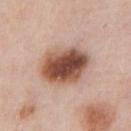Assessment:
No biopsy was performed on this lesion — it was imaged during a full skin examination and was not determined to be concerning.
Acquisition and patient details:
Captured under white-light illumination. The lesion's longest dimension is about 6 mm. Automated image analysis of the tile measured an eccentricity of roughly 0.65 and two-axis asymmetry of about 0.1. The analysis additionally found an automated nevus-likeness rating near 100 out of 100 and a detector confidence of about 100 out of 100 that the crop contains a lesion. Located on the front of the torso. A male patient in their mid- to late 50s. A 15 mm crop from a total-body photograph taken for skin-cancer surveillance.